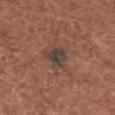Context:
Imaged with white-light lighting. The lesion is located on the left thigh. A 15 mm close-up tile from a total-body photography series done for melanoma screening. A male patient, aged around 75.